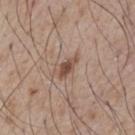Q: Was this lesion biopsied?
A: no biopsy performed (imaged during a skin exam)
Q: What is the imaging modality?
A: 15 mm crop, total-body photography
Q: What lighting was used for the tile?
A: white-light
Q: Automated lesion metrics?
A: a border-irregularity rating of about 3/10 and a color-variation rating of about 1.5/10
Q: Where on the body is the lesion?
A: the chest
Q: How large is the lesion?
A: ~3 mm (longest diameter)
Q: What are the patient's age and sex?
A: male, aged 73–77